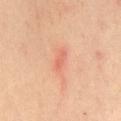biopsy status = imaged on a skin check; not biopsied | lesion diameter = ~3 mm (longest diameter) | acquisition = 15 mm crop, total-body photography | body site = the mid back | image-analysis metrics = a lesion color around L≈66 a*≈30 b*≈33 in CIELAB, roughly 8 lightness units darker than nearby skin, and a normalized border contrast of about 4.5; a border-irregularity index near 3.5/10, a within-lesion color-variation index near 1.5/10, and a peripheral color-asymmetry measure near 0.5; a detector confidence of about 100 out of 100 that the crop contains a lesion | illumination = cross-polarized | patient = female, about 40 years old.